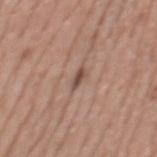Clinical impression: Captured during whole-body skin photography for melanoma surveillance; the lesion was not biopsied. Image and clinical context: This is a white-light tile. A male subject in their mid-50s. A 15 mm close-up extracted from a 3D total-body photography capture. Approximately 2.5 mm at its widest. The lesion is located on the mid back.